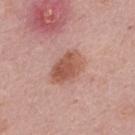Imaged during a routine full-body skin examination; the lesion was not biopsied and no histopathology is available.